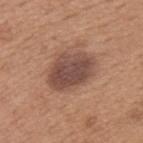notes: no biopsy performed (imaged during a skin exam) | subject: male, about 65 years old | lighting: white-light illumination | lesion diameter: ~5.5 mm (longest diameter) | image source: total-body-photography crop, ~15 mm field of view | automated lesion analysis: an area of roughly 16 mm², a shape eccentricity near 0.75, and two-axis asymmetry of about 0.2; a lesion color around L≈47 a*≈19 b*≈25 in CIELAB, roughly 12 lightness units darker than nearby skin, and a normalized border contrast of about 10; a detector confidence of about 100 out of 100 that the crop contains a lesion | body site: the upper back.